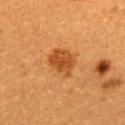Case summary:
* workup: total-body-photography surveillance lesion; no biopsy
* site: the upper back
* lesion size: about 3.5 mm
* acquisition: ~15 mm tile from a whole-body skin photo
* subject: female, about 55 years old
* illumination: cross-polarized illumination